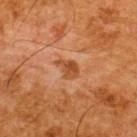Part of a total-body skin-imaging series; this lesion was reviewed on a skin check and was not flagged for biopsy.
A male patient approximately 65 years of age.
Located on the upper back.
A 15 mm close-up tile from a total-body photography series done for melanoma screening.
The tile uses cross-polarized illumination.